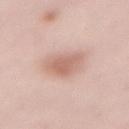Clinical impression: Captured during whole-body skin photography for melanoma surveillance; the lesion was not biopsied. Context: A female patient, about 50 years old. A region of skin cropped from a whole-body photographic capture, roughly 15 mm wide. Approximately 3.5 mm at its widest. On the abdomen.